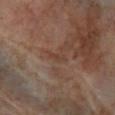– follow-up — total-body-photography surveillance lesion; no biopsy
– patient — male, aged 68 to 72
– site — the right lower leg
– imaging modality — ~15 mm tile from a whole-body skin photo
– automated metrics — an area of roughly 2.5 mm², an eccentricity of roughly 0.9, and a shape-asymmetry score of about 0.4 (0 = symmetric); a lesion color around L≈39 a*≈17 b*≈26 in CIELAB, roughly 5 lightness units darker than nearby skin, and a normalized lesion–skin contrast near 5.5; a nevus-likeness score of about 0/100 and a lesion-detection confidence of about 80/100
– lighting — cross-polarized illumination
– lesion diameter — about 2.5 mm